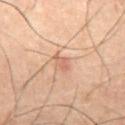notes — imaged on a skin check; not biopsied | image source — ~15 mm tile from a whole-body skin photo | tile lighting — cross-polarized illumination | subject — male, aged 58–62 | location — the abdomen | size — ~2.5 mm (longest diameter).